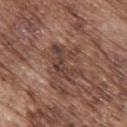Part of a total-body skin-imaging series; this lesion was reviewed on a skin check and was not flagged for biopsy.
The lesion is located on the upper back.
A 15 mm close-up extracted from a 3D total-body photography capture.
Approximately 5 mm at its widest.
The patient is a male roughly 75 years of age.
This is a white-light tile.